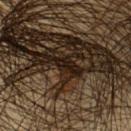Case summary:
* notes: total-body-photography surveillance lesion; no biopsy
* site: the left upper arm
* tile lighting: cross-polarized
* diameter: ~3 mm (longest diameter)
* automated metrics: an average lesion color of about L≈16 a*≈12 b*≈18 (CIELAB), about 10 CIELAB-L* units darker than the surrounding skin, and a normalized lesion–skin contrast near 13.5
* subject: male, approximately 45 years of age
* image: 15 mm crop, total-body photography